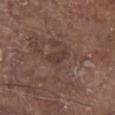Assessment:
Imaged during a routine full-body skin examination; the lesion was not biopsied and no histopathology is available.
Context:
On the chest. A 15 mm close-up tile from a total-body photography series done for melanoma screening. The patient is a male aged around 80. This is a white-light tile. The lesion-visualizer software estimated a footprint of about 3 mm², an eccentricity of roughly 0.85, and a shape-asymmetry score of about 0.3 (0 = symmetric). Measured at roughly 2.5 mm in maximum diameter.